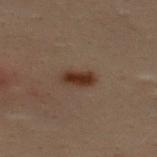The lesion was tiled from a total-body skin photograph and was not biopsied. The lesion is on the upper back. A male patient aged approximately 30. An algorithmic analysis of the crop reported a lesion color around L≈26 a*≈15 b*≈22 in CIELAB, about 9 CIELAB-L* units darker than the surrounding skin, and a lesion-to-skin contrast of about 10.5 (normalized; higher = more distinct). The software also gave a classifier nevus-likeness of about 100/100 and a lesion-detection confidence of about 100/100. A 15 mm crop from a total-body photograph taken for skin-cancer surveillance. Measured at roughly 3.5 mm in maximum diameter.